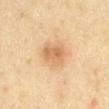Clinical impression:
Recorded during total-body skin imaging; not selected for excision or biopsy.
Acquisition and patient details:
The total-body-photography lesion software estimated a footprint of about 6.5 mm² and two-axis asymmetry of about 0.15. It also reported a border-irregularity index near 2/10 and radial color variation of about 1. It also reported an automated nevus-likeness rating near 75 out of 100. Captured under cross-polarized illumination. Cropped from a whole-body photographic skin survey; the tile spans about 15 mm. Longest diameter approximately 3 mm. A male patient about 45 years old. The lesion is located on the mid back.